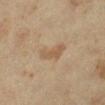tile lighting=cross-polarized; size=about 4 mm; patient=female, aged around 40; body site=the left lower leg; imaging modality=~15 mm crop, total-body skin-cancer survey.